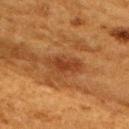notes=imaged on a skin check; not biopsied | diameter=≈4.5 mm | subject=female, aged around 50 | tile lighting=cross-polarized illumination | location=the upper back | image source=~15 mm crop, total-body skin-cancer survey.